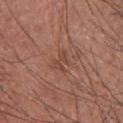This lesion was catalogued during total-body skin photography and was not selected for biopsy. The lesion is located on the chest. The recorded lesion diameter is about 2.5 mm. A male subject about 65 years old. This is a white-light tile. A close-up tile cropped from a whole-body skin photograph, about 15 mm across.